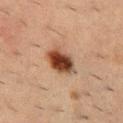Clinical impression: Captured during whole-body skin photography for melanoma surveillance; the lesion was not biopsied.